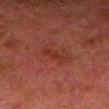<lesion>
<biopsy_status>not biopsied; imaged during a skin examination</biopsy_status>
<site>right lower leg</site>
<patient>
  <sex>male</sex>
  <age_approx>80</age_approx>
</patient>
<image>
  <source>total-body photography crop</source>
  <field_of_view_mm>15</field_of_view_mm>
</image>
</lesion>